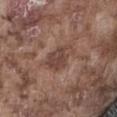No biopsy was performed on this lesion — it was imaged during a full skin examination and was not determined to be concerning.
The lesion's longest dimension is about 3.5 mm.
Imaged with white-light lighting.
An algorithmic analysis of the crop reported about 9 CIELAB-L* units darker than the surrounding skin and a normalized lesion–skin contrast near 7. And it measured a nevus-likeness score of about 0/100 and a detector confidence of about 100 out of 100 that the crop contains a lesion.
The patient is a male aged approximately 75.
On the abdomen.
A lesion tile, about 15 mm wide, cut from a 3D total-body photograph.